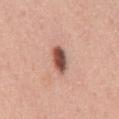| key | value |
|---|---|
| biopsy status | no biopsy performed (imaged during a skin exam) |
| image-analysis metrics | an automated nevus-likeness rating near 95 out of 100 and a lesion-detection confidence of about 100/100 |
| patient | male, roughly 60 years of age |
| illumination | white-light illumination |
| image | ~15 mm tile from a whole-body skin photo |
| location | the mid back |